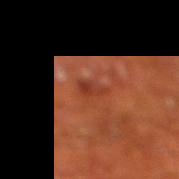| feature | finding |
|---|---|
| patient | male, aged approximately 65 |
| lesion diameter | ≈2.5 mm |
| automated lesion analysis | a footprint of about 2 mm² and a shape eccentricity near 0.9; border irregularity of about 5 on a 0–10 scale and radial color variation of about 0; a classifier nevus-likeness of about 0/100 and lesion-presence confidence of about 100/100 |
| location | the right lower leg |
| image | ~15 mm tile from a whole-body skin photo |
| illumination | cross-polarized |